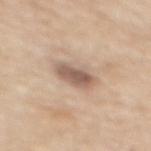biopsy status: total-body-photography surveillance lesion; no biopsy